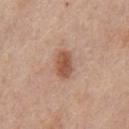{
  "biopsy_status": "not biopsied; imaged during a skin examination",
  "lesion_size": {
    "long_diameter_mm_approx": 3.5
  },
  "patient": {
    "sex": "male",
    "age_approx": 75
  },
  "automated_metrics": {
    "area_mm2_approx": 6.5,
    "eccentricity": 0.8,
    "shape_asymmetry": 0.25,
    "border_irregularity_0_10": 2.5,
    "color_variation_0_10": 3.0,
    "peripheral_color_asymmetry": 1.0
  },
  "image": {
    "source": "total-body photography crop",
    "field_of_view_mm": 15
  },
  "site": "front of the torso"
}